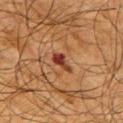Recorded during total-body skin imaging; not selected for excision or biopsy. Cropped from a whole-body photographic skin survey; the tile spans about 15 mm. The subject is a male aged around 65. The recorded lesion diameter is about 3 mm. The lesion-visualizer software estimated a border-irregularity index near 4/10, internal color variation of about 1.5 on a 0–10 scale, and peripheral color asymmetry of about 0.5. On the right upper arm.